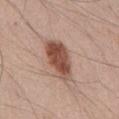Imaged during a routine full-body skin examination; the lesion was not biopsied and no histopathology is available. A male subject aged approximately 45. The tile uses white-light illumination. A roughly 15 mm field-of-view crop from a total-body skin photograph. The total-body-photography lesion software estimated a mean CIELAB color near L≈50 a*≈21 b*≈27 and a lesion–skin lightness drop of about 15. The lesion is located on the abdomen. The recorded lesion diameter is about 5.5 mm.